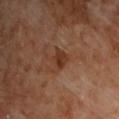Impression:
Imaged during a routine full-body skin examination; the lesion was not biopsied and no histopathology is available.
Image and clinical context:
A lesion tile, about 15 mm wide, cut from a 3D total-body photograph. Measured at roughly 2.5 mm in maximum diameter. A male subject aged approximately 60. The lesion is on the upper back.